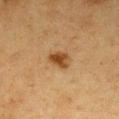notes: imaged on a skin check; not biopsied | image: ~15 mm tile from a whole-body skin photo | site: the chest | lighting: cross-polarized | lesion size: about 3 mm | subject: female, aged approximately 40.